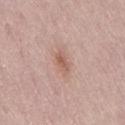This lesion was catalogued during total-body skin photography and was not selected for biopsy.